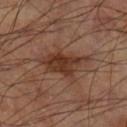Captured during whole-body skin photography for melanoma surveillance; the lesion was not biopsied. From the right thigh. Imaged with cross-polarized lighting. Approximately 6 mm at its widest. A close-up tile cropped from a whole-body skin photograph, about 15 mm across.